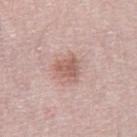follow-up — total-body-photography surveillance lesion; no biopsy
site — the leg
lesion size — ≈3.5 mm
TBP lesion metrics — a symmetry-axis asymmetry near 0.35; a lesion–skin lightness drop of about 10
subject — female, roughly 55 years of age
illumination — white-light
acquisition — 15 mm crop, total-body photography